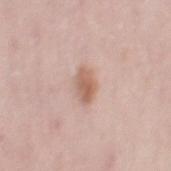  site: right thigh
  lighting: white-light
  image:
    source: total-body photography crop
    field_of_view_mm: 15
  patient:
    sex: female
    age_approx: 30